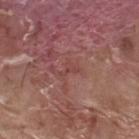The lesion was tiled from a total-body skin photograph and was not biopsied.
On the upper back.
Cropped from a total-body skin-imaging series; the visible field is about 15 mm.
The subject is a male roughly 70 years of age.
About 2.5 mm across.
The tile uses white-light illumination.